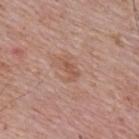| feature | finding |
|---|---|
| biopsy status | total-body-photography surveillance lesion; no biopsy |
| imaging modality | ~15 mm tile from a whole-body skin photo |
| subject | male, aged 63–67 |
| anatomic site | the upper back |